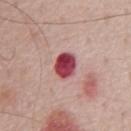The lesion was tiled from a total-body skin photograph and was not biopsied. On the chest. A male subject aged 68–72. Measured at roughly 3 mm in maximum diameter. A 15 mm crop from a total-body photograph taken for skin-cancer surveillance. The tile uses white-light illumination. An algorithmic analysis of the crop reported a border-irregularity rating of about 1/10 and a within-lesion color-variation index near 6.5/10. The analysis additionally found a classifier nevus-likeness of about 0/100 and a detector confidence of about 100 out of 100 that the crop contains a lesion.